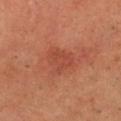{
  "lesion_size": {
    "long_diameter_mm_approx": 4.0
  },
  "image": {
    "source": "total-body photography crop",
    "field_of_view_mm": 15
  },
  "patient": {
    "sex": "male",
    "age_approx": 55
  },
  "automated_metrics": {
    "eccentricity": 0.75,
    "shape_asymmetry": 0.35
  },
  "lighting": "cross-polarized",
  "site": "head or neck"
}